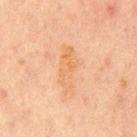* biopsy status — imaged on a skin check; not biopsied
* body site — the chest
* tile lighting — cross-polarized
* size — ≈5.5 mm
* imaging modality — total-body-photography crop, ~15 mm field of view
* patient — male, in their mid-40s
* automated lesion analysis — a footprint of about 10 mm², a shape eccentricity near 0.9, and a shape-asymmetry score of about 0.35 (0 = symmetric); internal color variation of about 3.5 on a 0–10 scale and peripheral color asymmetry of about 1.5